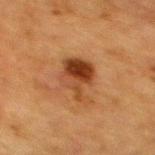Findings:
– image · total-body-photography crop, ~15 mm field of view
– TBP lesion metrics · a mean CIELAB color near L≈35 a*≈22 b*≈32 and a lesion–skin lightness drop of about 11
– anatomic site · the upper back
– lighting · cross-polarized illumination
– subject · female, aged 53–57
– size · ~4.5 mm (longest diameter)